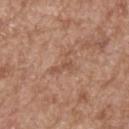workup = imaged on a skin check; not biopsied
image = 15 mm crop, total-body photography
site = the upper back
lesion size = ~3.5 mm (longest diameter)
subject = male, approximately 65 years of age
image-analysis metrics = a lesion color around L≈52 a*≈21 b*≈29 in CIELAB; a border-irregularity index near 8/10 and internal color variation of about 0.5 on a 0–10 scale; lesion-presence confidence of about 100/100